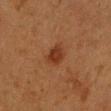The lesion was photographed on a routine skin check and not biopsied; there is no pathology result. Imaged with cross-polarized lighting. Longest diameter approximately 2.5 mm. Located on the right forearm. A lesion tile, about 15 mm wide, cut from a 3D total-body photograph. A female patient, about 55 years old.